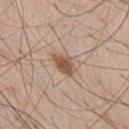The lesion was tiled from a total-body skin photograph and was not biopsied.
On the chest.
A lesion tile, about 15 mm wide, cut from a 3D total-body photograph.
The total-body-photography lesion software estimated an eccentricity of roughly 0.8. And it measured a lesion color around L≈53 a*≈19 b*≈29 in CIELAB and roughly 12 lightness units darker than nearby skin. The software also gave a within-lesion color-variation index near 2/10 and radial color variation of about 0.5.
Captured under white-light illumination.
A male subject, aged approximately 60.